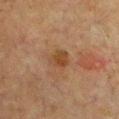| field | value |
|---|---|
| notes | imaged on a skin check; not biopsied |
| image source | total-body-photography crop, ~15 mm field of view |
| patient | male, aged 63–67 |
| anatomic site | the chest |